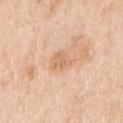workup: catalogued during a skin exam; not biopsied
illumination: white-light illumination
patient: male, aged approximately 70
site: the right upper arm
diameter: ~3 mm (longest diameter)
image: 15 mm crop, total-body photography
image-analysis metrics: a lesion area of about 5 mm², a shape eccentricity near 0.5, and a shape-asymmetry score of about 0.25 (0 = symmetric); a mean CIELAB color near L≈68 a*≈21 b*≈35 and a normalized lesion–skin contrast near 5; a border-irregularity rating of about 2.5/10; a classifier nevus-likeness of about 0/100 and a lesion-detection confidence of about 100/100